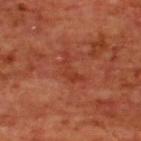Impression:
The lesion was tiled from a total-body skin photograph and was not biopsied.
Image and clinical context:
The lesion is located on the upper back. Automated image analysis of the tile measured a border-irregularity index near 7.5/10, a color-variation rating of about 1/10, and a peripheral color-asymmetry measure near 0. The software also gave a nevus-likeness score of about 0/100 and a detector confidence of about 100 out of 100 that the crop contains a lesion. About 4 mm across. Imaged with cross-polarized lighting. A male subject in their mid- to late 60s. A 15 mm close-up tile from a total-body photography series done for melanoma screening.